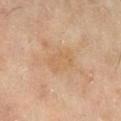Clinical summary:
The patient is a female in their 70s. A 15 mm close-up tile from a total-body photography series done for melanoma screening. The lesion is on the right lower leg. The tile uses cross-polarized illumination.